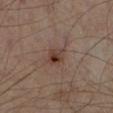biopsy status: catalogued during a skin exam; not biopsied
acquisition: 15 mm crop, total-body photography
subject: aged 53–57
automated lesion analysis: a lesion area of about 4.5 mm² and an eccentricity of roughly 0.65
anatomic site: the left lower leg